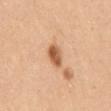Part of a total-body skin-imaging series; this lesion was reviewed on a skin check and was not flagged for biopsy.
Imaged with white-light lighting.
A female patient, aged 28–32.
An algorithmic analysis of the crop reported a within-lesion color-variation index near 4/10 and a peripheral color-asymmetry measure near 1.5.
The lesion is on the mid back.
A close-up tile cropped from a whole-body skin photograph, about 15 mm across.
The recorded lesion diameter is about 3 mm.